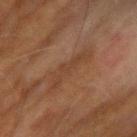workup: imaged on a skin check; not biopsied | lighting: cross-polarized illumination | imaging modality: 15 mm crop, total-body photography | diameter: ≈5.5 mm | image-analysis metrics: a normalized border contrast of about 5; border irregularity of about 8 on a 0–10 scale, internal color variation of about 0.5 on a 0–10 scale, and peripheral color asymmetry of about 0 | subject: male, in their 70s | body site: the right forearm.